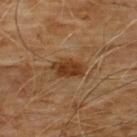Q: Is there a histopathology result?
A: catalogued during a skin exam; not biopsied
Q: What kind of image is this?
A: ~15 mm tile from a whole-body skin photo
Q: How large is the lesion?
A: about 4 mm
Q: Where on the body is the lesion?
A: the chest
Q: What lighting was used for the tile?
A: cross-polarized
Q: What are the patient's age and sex?
A: male, aged approximately 60